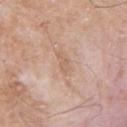biopsy status: no biopsy performed (imaged during a skin exam).